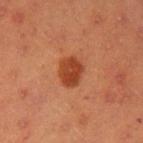<tbp_lesion>
  <biopsy_status>not biopsied; imaged during a skin examination</biopsy_status>
  <image>
    <source>total-body photography crop</source>
    <field_of_view_mm>15</field_of_view_mm>
  </image>
  <site>left upper arm</site>
  <patient>
    <sex>male</sex>
    <age_approx>40</age_approx>
  </patient>
</tbp_lesion>